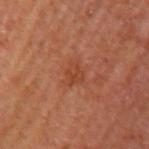Imaged during a routine full-body skin examination; the lesion was not biopsied and no histopathology is available.
A 15 mm close-up tile from a total-body photography series done for melanoma screening.
The patient is a male roughly 65 years of age.
Located on the arm.